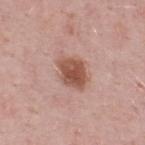{"biopsy_status": "not biopsied; imaged during a skin examination", "lighting": "white-light", "patient": {"sex": "male", "age_approx": 50}, "lesion_size": {"long_diameter_mm_approx": 4.5}, "site": "upper back", "image": {"source": "total-body photography crop", "field_of_view_mm": 15}}